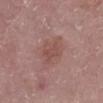Imaged during a routine full-body skin examination; the lesion was not biopsied and no histopathology is available. A roughly 15 mm field-of-view crop from a total-body skin photograph. About 4 mm across. A female subject, aged around 65. The tile uses white-light illumination. The total-body-photography lesion software estimated a nevus-likeness score of about 0/100 and a detector confidence of about 100 out of 100 that the crop contains a lesion. Located on the left lower leg.